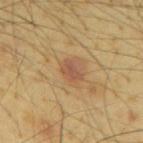Impression:
Part of a total-body skin-imaging series; this lesion was reviewed on a skin check and was not flagged for biopsy.
Image and clinical context:
Captured under cross-polarized illumination. The patient is a male aged 63–67. Cropped from a whole-body photographic skin survey; the tile spans about 15 mm. The lesion is on the mid back. The lesion's longest dimension is about 3 mm.